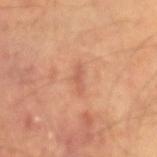follow-up: total-body-photography surveillance lesion; no biopsy
site: the left forearm
patient: male, about 60 years old
tile lighting: cross-polarized
image: ~15 mm tile from a whole-body skin photo